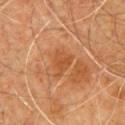The lesion was tiled from a total-body skin photograph and was not biopsied. This image is a 15 mm lesion crop taken from a total-body photograph. The total-body-photography lesion software estimated an average lesion color of about L≈47 a*≈25 b*≈38 (CIELAB), a lesion–skin lightness drop of about 7, and a normalized border contrast of about 6. It also reported a within-lesion color-variation index near 3/10 and radial color variation of about 1. The analysis additionally found a classifier nevus-likeness of about 0/100 and lesion-presence confidence of about 100/100. A male subject, about 60 years old. The lesion is located on the chest. Imaged with cross-polarized lighting.